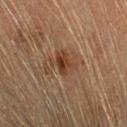biopsy_status: not biopsied; imaged during a skin examination
patient:
  sex: female
  age_approx: 40
site: head or neck
image:
  source: total-body photography crop
  field_of_view_mm: 15
lesion_size:
  long_diameter_mm_approx: 3.0
lighting: cross-polarized